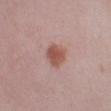Clinical impression:
The lesion was tiled from a total-body skin photograph and was not biopsied.
Image and clinical context:
Captured under white-light illumination. The lesion is located on the leg. The subject is a female aged around 40. Longest diameter approximately 3 mm. The lesion-visualizer software estimated a mean CIELAB color near L≈53 a*≈23 b*≈26. The analysis additionally found peripheral color asymmetry of about 0.5. A region of skin cropped from a whole-body photographic capture, roughly 15 mm wide.